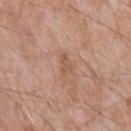Case summary:
* follow-up · catalogued during a skin exam; not biopsied
* automated metrics · a lesion area of about 3 mm², an outline eccentricity of about 0.8 (0 = round, 1 = elongated), and a symmetry-axis asymmetry near 0.45
* acquisition · 15 mm crop, total-body photography
* lighting · white-light
* body site · the mid back
* subject · male, aged 58–62
* lesion diameter · about 2.5 mm